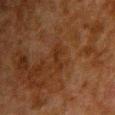biopsy status: total-body-photography surveillance lesion; no biopsy
illumination: cross-polarized illumination
subject: male, aged 58–62
lesion size: ≈3 mm
acquisition: ~15 mm tile from a whole-body skin photo
location: the chest
image-analysis metrics: a footprint of about 3 mm², an eccentricity of roughly 0.9, and a symmetry-axis asymmetry near 0.4